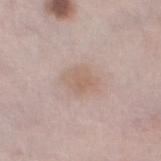  lesion_size:
    long_diameter_mm_approx: 3.0
  lighting: white-light
  automated_metrics:
    eccentricity: 0.65
    shape_asymmetry: 0.2
  image:
    source: total-body photography crop
    field_of_view_mm: 15
  site: left thigh
  patient:
    sex: male
    age_approx: 65